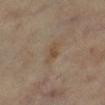| key | value |
|---|---|
| notes | imaged on a skin check; not biopsied |
| patient | female, in their mid-60s |
| automated lesion analysis | a lesion area of about 5 mm² and two-axis asymmetry of about 0.3; an average lesion color of about L≈47 a*≈13 b*≈28 (CIELAB), a lesion–skin lightness drop of about 6, and a lesion-to-skin contrast of about 5.5 (normalized; higher = more distinct); border irregularity of about 3 on a 0–10 scale, a within-lesion color-variation index near 2/10, and radial color variation of about 0.5 |
| anatomic site | the right lower leg |
| acquisition | 15 mm crop, total-body photography |
| size | ≈3.5 mm |